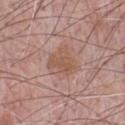Clinical impression:
Part of a total-body skin-imaging series; this lesion was reviewed on a skin check and was not flagged for biopsy.
Acquisition and patient details:
Longest diameter approximately 3.5 mm. A male patient approximately 70 years of age. An algorithmic analysis of the crop reported a shape eccentricity near 0.5 and two-axis asymmetry of about 0.3. The analysis additionally found an average lesion color of about L≈54 a*≈20 b*≈28 (CIELAB), roughly 7 lightness units darker than nearby skin, and a normalized lesion–skin contrast near 6.5. A lesion tile, about 15 mm wide, cut from a 3D total-body photograph. This is a white-light tile. On the chest.